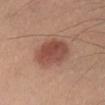Imaged during a routine full-body skin examination; the lesion was not biopsied and no histopathology is available.
This is a white-light tile.
Longest diameter approximately 5 mm.
On the chest.
This image is a 15 mm lesion crop taken from a total-body photograph.
A male patient aged 53 to 57.